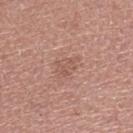biopsy status: no biopsy performed (imaged during a skin exam)
TBP lesion metrics: a footprint of about 4.5 mm², an eccentricity of roughly 0.7, and two-axis asymmetry of about 0.45; a mean CIELAB color near L≈55 a*≈21 b*≈26, about 7 CIELAB-L* units darker than the surrounding skin, and a lesion-to-skin contrast of about 4.5 (normalized; higher = more distinct)
site: the right lower leg
lesion size: about 3 mm
patient: female, aged around 50
imaging modality: ~15 mm tile from a whole-body skin photo
lighting: white-light